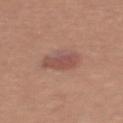Image and clinical context:
A roughly 15 mm field-of-view crop from a total-body skin photograph. From the chest. The tile uses white-light illumination. Automated tile analysis of the lesion measured about 9 CIELAB-L* units darker than the surrounding skin and a normalized border contrast of about 6.5. The analysis additionally found an automated nevus-likeness rating near 55 out of 100 and a detector confidence of about 100 out of 100 that the crop contains a lesion. The patient is a female roughly 35 years of age. The lesion's longest dimension is about 3.5 mm.